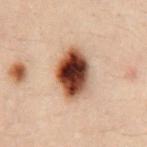Q: Was this lesion biopsied?
A: imaged on a skin check; not biopsied
Q: What did automated image analysis measure?
A: about 22 CIELAB-L* units darker than the surrounding skin and a lesion-to-skin contrast of about 16.5 (normalized; higher = more distinct); border irregularity of about 1.5 on a 0–10 scale and a peripheral color-asymmetry measure near 3.5
Q: Who is the patient?
A: male, approximately 30 years of age
Q: How large is the lesion?
A: ~5.5 mm (longest diameter)
Q: What is the anatomic site?
A: the chest
Q: What kind of image is this?
A: 15 mm crop, total-body photography